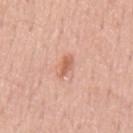From the mid back.
The recorded lesion diameter is about 2.5 mm.
A male patient aged 53–57.
An algorithmic analysis of the crop reported a footprint of about 3 mm² and a symmetry-axis asymmetry near 0.3. The software also gave a nevus-likeness score of about 35/100 and a detector confidence of about 100 out of 100 that the crop contains a lesion.
A 15 mm close-up tile from a total-body photography series done for melanoma screening.